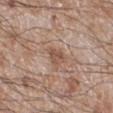The lesion was tiled from a total-body skin photograph and was not biopsied.
A male subject aged around 60.
Approximately 3 mm at its widest.
A close-up tile cropped from a whole-body skin photograph, about 15 mm across.
The lesion is on the right lower leg.